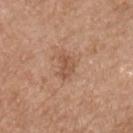Clinical impression:
This lesion was catalogued during total-body skin photography and was not selected for biopsy.
Clinical summary:
This image is a 15 mm lesion crop taken from a total-body photograph. Longest diameter approximately 3 mm. A male subject, about 65 years old. Located on the front of the torso. This is a white-light tile. An algorithmic analysis of the crop reported an area of roughly 4 mm² and two-axis asymmetry of about 0.35. It also reported a normalized border contrast of about 6. The analysis additionally found a classifier nevus-likeness of about 0/100 and lesion-presence confidence of about 100/100.